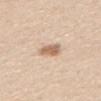Notes:
– biopsy status — total-body-photography surveillance lesion; no biopsy
– subject — female, about 60 years old
– image source — ~15 mm crop, total-body skin-cancer survey
– site — the upper back